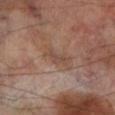Impression: Imaged during a routine full-body skin examination; the lesion was not biopsied and no histopathology is available. Acquisition and patient details: The lesion's longest dimension is about 3 mm. A male patient, in their 70s. From the leg. A 15 mm close-up tile from a total-body photography series done for melanoma screening.